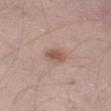Assessment: The lesion was photographed on a routine skin check and not biopsied; there is no pathology result. Image and clinical context: The lesion-visualizer software estimated a lesion area of about 4 mm² and a shape-asymmetry score of about 0.15 (0 = symmetric). And it measured a border-irregularity rating of about 1.5/10, a within-lesion color-variation index near 2/10, and radial color variation of about 1. The analysis additionally found a classifier nevus-likeness of about 75/100. A male patient, in their mid- to late 40s. On the left thigh. A region of skin cropped from a whole-body photographic capture, roughly 15 mm wide. Captured under white-light illumination. Approximately 3 mm at its widest.